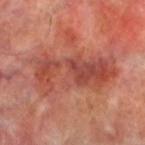Notes:
- follow-up — no biopsy performed (imaged during a skin exam)
- diameter — ~9.5 mm (longest diameter)
- image source — 15 mm crop, total-body photography
- anatomic site — the left lower leg
- illumination — cross-polarized illumination
- automated metrics — a lesion-to-skin contrast of about 6.5 (normalized; higher = more distinct); a border-irregularity index near 6/10, a within-lesion color-variation index near 6.5/10, and radial color variation of about 2
- patient — male, in their 70s